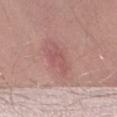<case>
<biopsy_status>not biopsied; imaged during a skin examination</biopsy_status>
<site>leg</site>
<patient>
  <sex>male</sex>
  <age_approx>40</age_approx>
</patient>
<automated_metrics>
  <area_mm2_approx>6.5</area_mm2_approx>
  <eccentricity>0.9</eccentricity>
  <shape_asymmetry>0.4</shape_asymmetry>
  <cielab_L>54</cielab_L>
  <cielab_a>25</cielab_a>
  <cielab_b>22</cielab_b>
  <vs_skin_contrast_norm>5.0</vs_skin_contrast_norm>
  <border_irregularity_0_10>5.0</border_irregularity_0_10>
  <peripheral_color_asymmetry>0.5</peripheral_color_asymmetry>
</automated_metrics>
<image>
  <source>total-body photography crop</source>
  <field_of_view_mm>15</field_of_view_mm>
</image>
</case>